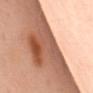Imaged during a routine full-body skin examination; the lesion was not biopsied and no histopathology is available.
The lesion is located on the mid back.
Automated image analysis of the tile measured an average lesion color of about L≈54 a*≈23 b*≈31 (CIELAB), roughly 17 lightness units darker than nearby skin, and a normalized lesion–skin contrast near 11. The analysis additionally found a classifier nevus-likeness of about 0/100.
Longest diameter approximately 11.5 mm.
A male patient, aged 53 to 57.
A roughly 15 mm field-of-view crop from a total-body skin photograph.